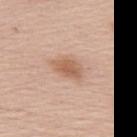No biopsy was performed on this lesion — it was imaged during a full skin examination and was not determined to be concerning.
A 15 mm crop from a total-body photograph taken for skin-cancer surveillance.
The total-body-photography lesion software estimated an area of roughly 7 mm² and two-axis asymmetry of about 0.25. The software also gave an average lesion color of about L≈60 a*≈20 b*≈31 (CIELAB), about 10 CIELAB-L* units darker than the surrounding skin, and a normalized border contrast of about 7.5.
From the upper back.
The subject is a female in their mid- to late 60s.
The tile uses white-light illumination.